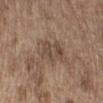<tbp_lesion>
  <biopsy_status>not biopsied; imaged during a skin examination</biopsy_status>
  <image>
    <source>total-body photography crop</source>
    <field_of_view_mm>15</field_of_view_mm>
  </image>
  <patient>
    <sex>male</sex>
    <age_approx>70</age_approx>
  </patient>
  <lighting>white-light</lighting>
  <site>right lower leg</site>
  <lesion_size>
    <long_diameter_mm_approx>5.0</long_diameter_mm_approx>
  </lesion_size>
</tbp_lesion>